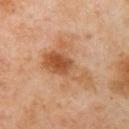Imaged during a routine full-body skin examination; the lesion was not biopsied and no histopathology is available.
Located on the left upper arm.
Measured at roughly 7 mm in maximum diameter.
Imaged with cross-polarized lighting.
The patient is a female in their 40s.
A region of skin cropped from a whole-body photographic capture, roughly 15 mm wide.